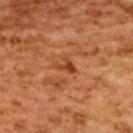<lesion>
<biopsy_status>not biopsied; imaged during a skin examination</biopsy_status>
<patient>
  <sex>female</sex>
  <age_approx>55</age_approx>
</patient>
<image>
  <source>total-body photography crop</source>
  <field_of_view_mm>15</field_of_view_mm>
</image>
<lesion_size>
  <long_diameter_mm_approx>2.5</long_diameter_mm_approx>
</lesion_size>
<site>upper back</site>
<automated_metrics>
  <area_mm2_approx>3.0</area_mm2_approx>
  <shape_asymmetry>0.55</shape_asymmetry>
  <cielab_L>46</cielab_L>
  <cielab_a>31</cielab_a>
  <cielab_b>43</cielab_b>
  <vs_skin_darker_L>10.0</vs_skin_darker_L>
  <vs_skin_contrast_norm>7.5</vs_skin_contrast_norm>
  <border_irregularity_0_10>5.5</border_irregularity_0_10>
  <color_variation_0_10>0.0</color_variation_0_10>
  <peripheral_color_asymmetry>0.0</peripheral_color_asymmetry>
  <nevus_likeness_0_100>0</nevus_likeness_0_100>
  <lesion_detection_confidence_0_100>100</lesion_detection_confidence_0_100>
</automated_metrics>
</lesion>